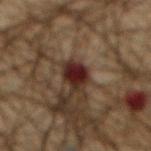Recorded during total-body skin imaging; not selected for excision or biopsy.
An algorithmic analysis of the crop reported an area of roughly 5.5 mm² and a shape eccentricity near 0.8. And it measured a border-irregularity rating of about 2.5/10, a color-variation rating of about 3.5/10, and a peripheral color-asymmetry measure near 1. It also reported a nevus-likeness score of about 0/100.
A 15 mm close-up extracted from a 3D total-body photography capture.
From the abdomen.
The patient is a male approximately 65 years of age.
The tile uses cross-polarized illumination.
The recorded lesion diameter is about 3.5 mm.